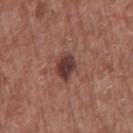notes — total-body-photography surveillance lesion; no biopsy | anatomic site — the chest | acquisition — total-body-photography crop, ~15 mm field of view | subject — male, roughly 75 years of age.